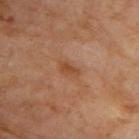notes = no biopsy performed (imaged during a skin exam); tile lighting = cross-polarized; patient = male, in their 70s; anatomic site = the upper back; acquisition = ~15 mm crop, total-body skin-cancer survey.